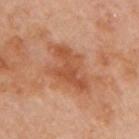No biopsy was performed on this lesion — it was imaged during a full skin examination and was not determined to be concerning.
The lesion is located on the arm.
A female subject in their 50s.
Captured under cross-polarized illumination.
The total-body-photography lesion software estimated a lesion color around L≈50 a*≈25 b*≈34 in CIELAB, a lesion–skin lightness drop of about 9, and a lesion-to-skin contrast of about 6.5 (normalized; higher = more distinct).
A roughly 15 mm field-of-view crop from a total-body skin photograph.
The lesion's longest dimension is about 6 mm.